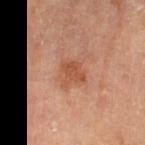<case>
<biopsy_status>not biopsied; imaged during a skin examination</biopsy_status>
<image>
  <source>total-body photography crop</source>
  <field_of_view_mm>15</field_of_view_mm>
</image>
<lesion_size>
  <long_diameter_mm_approx>2.5</long_diameter_mm_approx>
</lesion_size>
<automated_metrics>
  <nevus_likeness_0_100>20</nevus_likeness_0_100>
  <lesion_detection_confidence_0_100>100</lesion_detection_confidence_0_100>
</automated_metrics>
<site>left thigh</site>
<patient>
  <sex>male</sex>
  <age_approx>85+</age_approx>
</patient>
<lighting>cross-polarized</lighting>
</case>